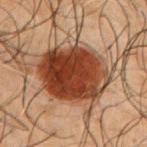{"biopsy_status": "not biopsied; imaged during a skin examination"}